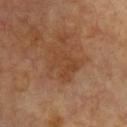Clinical impression: No biopsy was performed on this lesion — it was imaged during a full skin examination and was not determined to be concerning. Image and clinical context: A roughly 15 mm field-of-view crop from a total-body skin photograph. The subject is aged approximately 65. The lesion is on the arm. Measured at roughly 4 mm in maximum diameter.